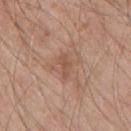| key | value |
|---|---|
| biopsy status | imaged on a skin check; not biopsied |
| lesion diameter | ≈3 mm |
| body site | the chest |
| patient | male, approximately 55 years of age |
| imaging modality | ~15 mm crop, total-body skin-cancer survey |